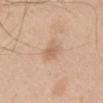{"biopsy_status": "not biopsied; imaged during a skin examination", "site": "chest", "patient": {"sex": "male", "age_approx": 60}, "automated_metrics": {"cielab_L": 63, "cielab_a": 19, "cielab_b": 33, "vs_skin_contrast_norm": 5.5}, "lighting": "white-light", "lesion_size": {"long_diameter_mm_approx": 2.5}, "image": {"source": "total-body photography crop", "field_of_view_mm": 15}}